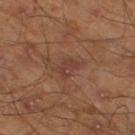workup: catalogued during a skin exam; not biopsied | subject: male, in their mid- to late 40s | imaging modality: total-body-photography crop, ~15 mm field of view | site: the left lower leg.